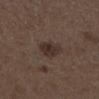Imaged during a routine full-body skin examination; the lesion was not biopsied and no histopathology is available. The subject is a male approximately 50 years of age. About 3 mm across. Cropped from a total-body skin-imaging series; the visible field is about 15 mm. The lesion is located on the left lower leg.